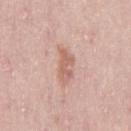Captured during whole-body skin photography for melanoma surveillance; the lesion was not biopsied.